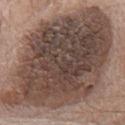{"biopsy_status": "not biopsied; imaged during a skin examination", "site": "mid back", "patient": {"sex": "male", "age_approx": 70}, "image": {"source": "total-body photography crop", "field_of_view_mm": 15}}